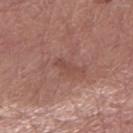Q: Was a biopsy performed?
A: imaged on a skin check; not biopsied
Q: What is the imaging modality?
A: ~15 mm crop, total-body skin-cancer survey
Q: Who is the patient?
A: male, approximately 60 years of age
Q: What lighting was used for the tile?
A: white-light
Q: Lesion location?
A: the arm
Q: What did automated image analysis measure?
A: a border-irregularity index near 3.5/10, internal color variation of about 0 on a 0–10 scale, and radial color variation of about 0